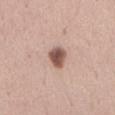workup — no biopsy performed (imaged during a skin exam)
size — about 3 mm
image — ~15 mm crop, total-body skin-cancer survey
lighting — white-light
location — the abdomen
automated metrics — an area of roughly 5.5 mm² and a symmetry-axis asymmetry near 0.2; a border-irregularity rating of about 2/10 and a peripheral color-asymmetry measure near 1; a classifier nevus-likeness of about 90/100 and a detector confidence of about 100 out of 100 that the crop contains a lesion
patient — female, aged 33–37